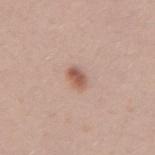notes: no biopsy performed (imaged during a skin exam)
site: the right upper arm
image source: 15 mm crop, total-body photography
TBP lesion metrics: an area of roughly 3.5 mm², an eccentricity of roughly 0.8, and two-axis asymmetry of about 0.25; a lesion–skin lightness drop of about 12 and a normalized lesion–skin contrast near 8.5; a detector confidence of about 100 out of 100 that the crop contains a lesion
patient: female, aged around 25
illumination: white-light
size: ~2.5 mm (longest diameter)